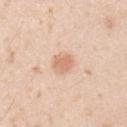Case summary:
* notes · catalogued during a skin exam; not biopsied
* diameter · about 2.5 mm
* subject · male, aged 23 to 27
* imaging modality · total-body-photography crop, ~15 mm field of view
* body site · the right upper arm
* tile lighting · white-light illumination